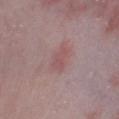Clinical impression: The lesion was tiled from a total-body skin photograph and was not biopsied.